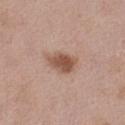biopsy_status: not biopsied; imaged during a skin examination
site: left thigh
lesion_size:
  long_diameter_mm_approx: 4.0
patient:
  sex: female
  age_approx: 40
image:
  source: total-body photography crop
  field_of_view_mm: 15